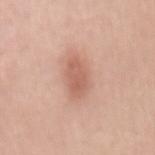lighting = white-light
image source = ~15 mm crop, total-body skin-cancer survey
location = the back
image-analysis metrics = a shape eccentricity near 0.9 and two-axis asymmetry of about 0.2; a border-irregularity rating of about 2.5/10, internal color variation of about 2 on a 0–10 scale, and radial color variation of about 0.5
patient = female, about 40 years old
diameter = ≈4.5 mm